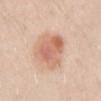Captured during whole-body skin photography for melanoma surveillance; the lesion was not biopsied. This image is a 15 mm lesion crop taken from a total-body photograph. This is a white-light tile. An algorithmic analysis of the crop reported an average lesion color of about L≈66 a*≈22 b*≈31 (CIELAB) and a normalized border contrast of about 7. It also reported an automated nevus-likeness rating near 95 out of 100 and lesion-presence confidence of about 100/100. The subject is a male about 30 years old. The lesion is located on the front of the torso.